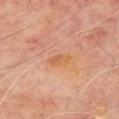<lesion>
<biopsy_status>not biopsied; imaged during a skin examination</biopsy_status>
<lesion_size>
  <long_diameter_mm_approx>3.0</long_diameter_mm_approx>
</lesion_size>
<lighting>cross-polarized</lighting>
<image>
  <source>total-body photography crop</source>
  <field_of_view_mm>15</field_of_view_mm>
</image>
<patient>
  <sex>male</sex>
  <age_approx>65</age_approx>
</patient>
<site>chest</site>
</lesion>